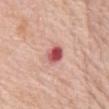Clinical summary: This is a white-light tile. Cropped from a total-body skin-imaging series; the visible field is about 15 mm. The lesion's longest dimension is about 3 mm. The lesion is on the abdomen. A female patient roughly 75 years of age.